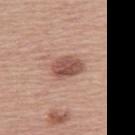The lesion was tiled from a total-body skin photograph and was not biopsied. A female patient, aged approximately 55. This is a white-light tile. This image is a 15 mm lesion crop taken from a total-body photograph. Located on the right thigh. The recorded lesion diameter is about 3.5 mm.